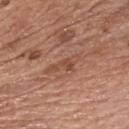Assessment:
The lesion was photographed on a routine skin check and not biopsied; there is no pathology result.
Clinical summary:
Cropped from a total-body skin-imaging series; the visible field is about 15 mm. The subject is a male aged approximately 70. An algorithmic analysis of the crop reported a mean CIELAB color near L≈49 a*≈23 b*≈31, roughly 7 lightness units darker than nearby skin, and a lesion-to-skin contrast of about 5.5 (normalized; higher = more distinct). And it measured border irregularity of about 5.5 on a 0–10 scale and internal color variation of about 2 on a 0–10 scale. The tile uses white-light illumination. The recorded lesion diameter is about 4.5 mm. Located on the front of the torso.